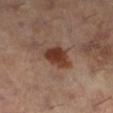Findings:
– notes: imaged on a skin check; not biopsied
– image source: total-body-photography crop, ~15 mm field of view
– automated lesion analysis: a footprint of about 8 mm², a shape eccentricity near 0.65, and two-axis asymmetry of about 0.25; a border-irregularity rating of about 2.5/10, internal color variation of about 4 on a 0–10 scale, and radial color variation of about 1; a classifier nevus-likeness of about 95/100 and a detector confidence of about 100 out of 100 that the crop contains a lesion
– lighting: cross-polarized illumination
– patient: female, aged 58–62
– anatomic site: the left lower leg
– diameter: ≈3.5 mm